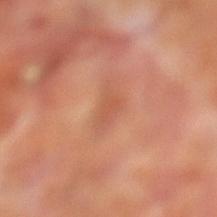Clinical impression: Imaged during a routine full-body skin examination; the lesion was not biopsied and no histopathology is available. Background: A male patient approximately 70 years of age. This image is a 15 mm lesion crop taken from a total-body photograph. The lesion is on the left lower leg. Automated tile analysis of the lesion measured a footprint of about 4 mm², an outline eccentricity of about 0.85 (0 = round, 1 = elongated), and a symmetry-axis asymmetry near 0.45. It also reported a lesion color around L≈54 a*≈26 b*≈33 in CIELAB, about 6 CIELAB-L* units darker than the surrounding skin, and a normalized lesion–skin contrast near 4.5. Approximately 3.5 mm at its widest. Imaged with cross-polarized lighting.